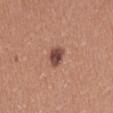Clinical impression: The lesion was tiled from a total-body skin photograph and was not biopsied. Clinical summary: The total-body-photography lesion software estimated an area of roughly 5 mm², an outline eccentricity of about 0.7 (0 = round, 1 = elongated), and two-axis asymmetry of about 0.3. The software also gave a mean CIELAB color near L≈46 a*≈22 b*≈25, roughly 14 lightness units darker than nearby skin, and a normalized lesion–skin contrast near 10.5. It also reported a border-irregularity rating of about 2.5/10, a color-variation rating of about 3.5/10, and peripheral color asymmetry of about 1. And it measured a classifier nevus-likeness of about 90/100 and lesion-presence confidence of about 100/100. A female patient about 40 years old. Imaged with white-light lighting. Located on the lower back. Approximately 3 mm at its widest. A 15 mm close-up extracted from a 3D total-body photography capture.